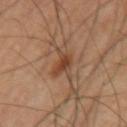A 15 mm crop from a total-body photograph taken for skin-cancer surveillance. About 5 mm across. The total-body-photography lesion software estimated a footprint of about 7.5 mm², an eccentricity of roughly 0.9, and two-axis asymmetry of about 0.5. The analysis additionally found a lesion color around L≈43 a*≈19 b*≈30 in CIELAB, about 10 CIELAB-L* units darker than the surrounding skin, and a normalized lesion–skin contrast near 8. The analysis additionally found a border-irregularity index near 7/10, internal color variation of about 4 on a 0–10 scale, and peripheral color asymmetry of about 1.5. A male patient aged approximately 60. Captured under cross-polarized illumination. On the left upper arm.